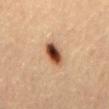Q: Was this lesion biopsied?
A: catalogued during a skin exam; not biopsied